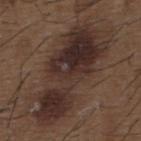Case summary:
- workup · total-body-photography surveillance lesion; no biopsy
- acquisition · ~15 mm crop, total-body skin-cancer survey
- lesion size · ≈13 mm
- automated lesion analysis · a mean CIELAB color near L≈30 a*≈15 b*≈20, about 10 CIELAB-L* units darker than the surrounding skin, and a normalized border contrast of about 10; internal color variation of about 8 on a 0–10 scale and a peripheral color-asymmetry measure near 3; an automated nevus-likeness rating near 20 out of 100
- subject · male, in their 50s
- lighting · white-light illumination
- location · the upper back